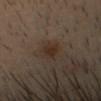Image and clinical context: The patient is a male aged approximately 30. This is a cross-polarized tile. A lesion tile, about 15 mm wide, cut from a 3D total-body photograph. Approximately 2.5 mm at its widest. The lesion is on the head or neck.